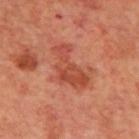illumination — cross-polarized | patient — male, about 70 years old | image — 15 mm crop, total-body photography | automated metrics — a lesion area of about 11 mm², an outline eccentricity of about 0.85 (0 = round, 1 = elongated), and two-axis asymmetry of about 0.55; a border-irregularity index near 7/10; a nevus-likeness score of about 0/100 | lesion diameter — about 5.5 mm | location — the mid back.